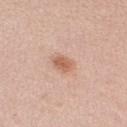Recorded during total-body skin imaging; not selected for excision or biopsy. A female subject roughly 35 years of age. This image is a 15 mm lesion crop taken from a total-body photograph. Imaged with white-light lighting. Measured at roughly 2.5 mm in maximum diameter. From the right upper arm.